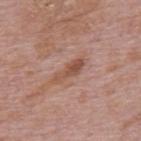Q: Automated lesion metrics?
A: a lesion area of about 5.5 mm², an outline eccentricity of about 0.95 (0 = round, 1 = elongated), and a symmetry-axis asymmetry near 0.25; a nevus-likeness score of about 0/100 and a detector confidence of about 100 out of 100 that the crop contains a lesion
Q: What is the lesion's diameter?
A: about 4.5 mm
Q: Who is the patient?
A: male, aged 73–77
Q: What is the anatomic site?
A: the mid back
Q: What is the imaging modality?
A: ~15 mm crop, total-body skin-cancer survey